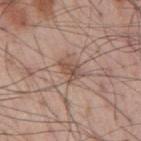The lesion was photographed on a routine skin check and not biopsied; there is no pathology result. The lesion is on the mid back. A male patient aged approximately 55. This is a white-light tile. Longest diameter approximately 3 mm. A 15 mm crop from a total-body photograph taken for skin-cancer surveillance.